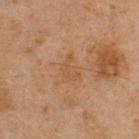– biopsy status — no biopsy performed (imaged during a skin exam)
– image — total-body-photography crop, ~15 mm field of view
– subject — male, about 45 years old
– automated metrics — a lesion area of about 3 mm², a shape eccentricity near 0.8, and a symmetry-axis asymmetry near 0.65; a lesion color around L≈42 a*≈17 b*≈30 in CIELAB and roughly 4 lightness units darker than nearby skin; a classifier nevus-likeness of about 0/100 and lesion-presence confidence of about 100/100
– tile lighting — cross-polarized
– size — ~3 mm (longest diameter)
– location — the upper back